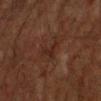The lesion was tiled from a total-body skin photograph and was not biopsied.
Approximately 4 mm at its widest.
A male subject, aged around 75.
A region of skin cropped from a whole-body photographic capture, roughly 15 mm wide.
Located on the right upper arm.
The tile uses cross-polarized illumination.